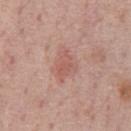workup — imaged on a skin check; not biopsied | location — the chest | image — 15 mm crop, total-body photography | subject — male, aged around 75.